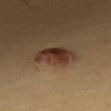anatomic site: the chest
image: total-body-photography crop, ~15 mm field of view
subject: male, in their 40s